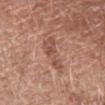The lesion was photographed on a routine skin check and not biopsied; there is no pathology result.
The patient is a female approximately 60 years of age.
A roughly 15 mm field-of-view crop from a total-body skin photograph.
From the right forearm.